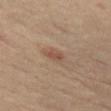Clinical impression:
Recorded during total-body skin imaging; not selected for excision or biopsy.
Background:
The lesion's longest dimension is about 2.5 mm. Cropped from a whole-body photographic skin survey; the tile spans about 15 mm. On the left thigh. This is a cross-polarized tile. The patient is a male aged 58 to 62.